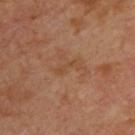No biopsy was performed on this lesion — it was imaged during a full skin examination and was not determined to be concerning. The recorded lesion diameter is about 3 mm. A patient approximately 65 years of age. Imaged with cross-polarized lighting. This image is a 15 mm lesion crop taken from a total-body photograph. An algorithmic analysis of the crop reported a lesion area of about 2 mm² and a shape-asymmetry score of about 0.6 (0 = symmetric). From the upper back.